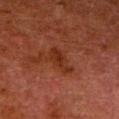Clinical impression: This lesion was catalogued during total-body skin photography and was not selected for biopsy. Context: The subject is a male about 80 years old. Approximately 4 mm at its widest. An algorithmic analysis of the crop reported a mean CIELAB color near L≈24 a*≈22 b*≈26, about 6 CIELAB-L* units darker than the surrounding skin, and a lesion-to-skin contrast of about 7 (normalized; higher = more distinct). The software also gave a lesion-detection confidence of about 100/100. The lesion is on the right lower leg. A 15 mm close-up tile from a total-body photography series done for melanoma screening. This is a cross-polarized tile.